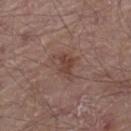<record>
<biopsy_status>not biopsied; imaged during a skin examination</biopsy_status>
<automated_metrics>
  <area_mm2_approx>5.0</area_mm2_approx>
  <eccentricity>0.8</eccentricity>
  <shape_asymmetry>0.4</shape_asymmetry>
  <cielab_L>42</cielab_L>
  <cielab_a>18</cielab_a>
  <cielab_b>24</cielab_b>
  <vs_skin_darker_L>8.0</vs_skin_darker_L>
  <vs_skin_contrast_norm>7.0</vs_skin_contrast_norm>
  <border_irregularity_0_10>5.0</border_irregularity_0_10>
  <color_variation_0_10>2.5</color_variation_0_10>
  <peripheral_color_asymmetry>1.0</peripheral_color_asymmetry>
</automated_metrics>
<lesion_size>
  <long_diameter_mm_approx>3.5</long_diameter_mm_approx>
</lesion_size>
<image>
  <source>total-body photography crop</source>
  <field_of_view_mm>15</field_of_view_mm>
</image>
<site>leg</site>
<lighting>white-light</lighting>
<patient>
  <sex>male</sex>
  <age_approx>80</age_approx>
</patient>
</record>